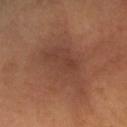This lesion was catalogued during total-body skin photography and was not selected for biopsy.
Captured under cross-polarized illumination.
On the left arm.
A lesion tile, about 15 mm wide, cut from a 3D total-body photograph.
The subject is a female aged around 45.
About 8 mm across.